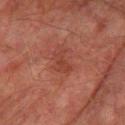Clinical impression: Imaged during a routine full-body skin examination; the lesion was not biopsied and no histopathology is available. Image and clinical context: A roughly 15 mm field-of-view crop from a total-body skin photograph. A male subject approximately 75 years of age. Located on the right thigh. The recorded lesion diameter is about 3 mm.